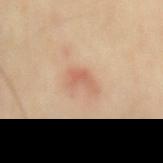{"biopsy_status": "not biopsied; imaged during a skin examination", "lesion_size": {"long_diameter_mm_approx": 3.5}, "site": "back", "lighting": "cross-polarized", "image": {"source": "total-body photography crop", "field_of_view_mm": 15}, "automated_metrics": {"vs_skin_darker_L": 9.0, "vs_skin_contrast_norm": 5.5, "border_irregularity_0_10": 4.0, "color_variation_0_10": 3.0, "peripheral_color_asymmetry": 1.0, "nevus_likeness_0_100": 40}, "patient": {"sex": "male", "age_approx": 65}}